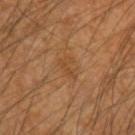Assessment: Part of a total-body skin-imaging series; this lesion was reviewed on a skin check and was not flagged for biopsy. Context: The lesion is on the left forearm. Captured under cross-polarized illumination. The patient is a male aged 58–62. A 15 mm close-up tile from a total-body photography series done for melanoma screening.